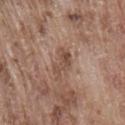biopsy_status: not biopsied; imaged during a skin examination
automated_metrics:
  cielab_L: 49
  cielab_a: 18
  cielab_b: 26
  vs_skin_darker_L: 9.0
  vs_skin_contrast_norm: 7.0
  color_variation_0_10: 3.0
  peripheral_color_asymmetry: 1.0
site: lower back
image:
  source: total-body photography crop
  field_of_view_mm: 15
patient:
  sex: male
  age_approx: 75